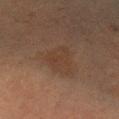Assessment:
This lesion was catalogued during total-body skin photography and was not selected for biopsy.
Background:
An algorithmic analysis of the crop reported a lesion area of about 9 mm², an eccentricity of roughly 0.65, and two-axis asymmetry of about 0.35. It also reported a classifier nevus-likeness of about 5/100 and a detector confidence of about 100 out of 100 that the crop contains a lesion. On the arm. This image is a 15 mm lesion crop taken from a total-body photograph. A female subject aged 53 to 57. Captured under cross-polarized illumination. The lesion's longest dimension is about 4 mm.